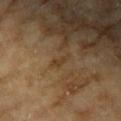Impression: Recorded during total-body skin imaging; not selected for excision or biopsy. Acquisition and patient details: The lesion is located on the arm. About 2.5 mm across. Automated tile analysis of the lesion measured a lesion area of about 2 mm², an eccentricity of roughly 0.95, and two-axis asymmetry of about 0.45. And it measured a border-irregularity index near 5/10. And it measured a detector confidence of about 75 out of 100 that the crop contains a lesion. A female subject aged around 60. A 15 mm close-up extracted from a 3D total-body photography capture.